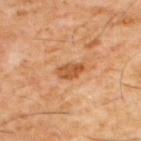Part of a total-body skin-imaging series; this lesion was reviewed on a skin check and was not flagged for biopsy.
Located on the back.
The subject is a male in their 60s.
A region of skin cropped from a whole-body photographic capture, roughly 15 mm wide.